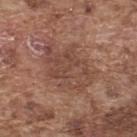Impression: The lesion was photographed on a routine skin check and not biopsied; there is no pathology result. Clinical summary: A roughly 15 mm field-of-view crop from a total-body skin photograph. The patient is a male in their mid- to late 70s. On the back.